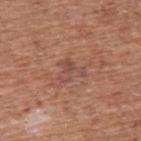<tbp_lesion>
  <biopsy_status>not biopsied; imaged during a skin examination</biopsy_status>
  <patient>
    <sex>male</sex>
    <age_approx>75</age_approx>
  </patient>
  <lesion_size>
    <long_diameter_mm_approx>3.0</long_diameter_mm_approx>
  </lesion_size>
  <image>
    <source>total-body photography crop</source>
    <field_of_view_mm>15</field_of_view_mm>
  </image>
  <automated_metrics>
    <area_mm2_approx>4.5</area_mm2_approx>
    <shape_asymmetry>0.7</shape_asymmetry>
    <cielab_L>48</cielab_L>
    <cielab_a>22</cielab_a>
    <cielab_b>26</cielab_b>
    <vs_skin_contrast_norm>5.5</vs_skin_contrast_norm>
    <color_variation_0_10>2.0</color_variation_0_10>
    <peripheral_color_asymmetry>0.5</peripheral_color_asymmetry>
  </automated_metrics>
  <lighting>white-light</lighting>
  <site>upper back</site>
</tbp_lesion>